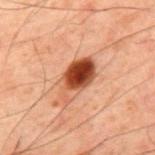Part of a total-body skin-imaging series; this lesion was reviewed on a skin check and was not flagged for biopsy. A 15 mm close-up tile from a total-body photography series done for melanoma screening. This is a cross-polarized tile. The lesion is located on the upper back. Longest diameter approximately 5 mm. The subject is a male aged 58 to 62.